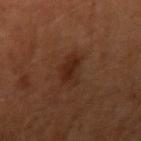{"biopsy_status": "not biopsied; imaged during a skin examination", "lighting": "cross-polarized", "lesion_size": {"long_diameter_mm_approx": 3.5}, "patient": {"sex": "male", "age_approx": 60}, "image": {"source": "total-body photography crop", "field_of_view_mm": 15}, "site": "right upper arm"}